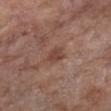No biopsy was performed on this lesion — it was imaged during a full skin examination and was not determined to be concerning.
From the chest.
Captured under white-light illumination.
A lesion tile, about 15 mm wide, cut from a 3D total-body photograph.
Automated image analysis of the tile measured a shape eccentricity near 0.65 and a shape-asymmetry score of about 0.25 (0 = symmetric). The software also gave an average lesion color of about L≈43 a*≈21 b*≈25 (CIELAB) and a lesion–skin lightness drop of about 8.
A female patient, aged 73–77.